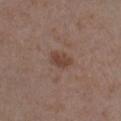- workup: catalogued during a skin exam; not biopsied
- lesion size: ≈2.5 mm
- subject: male, aged around 55
- image: ~15 mm crop, total-body skin-cancer survey
- site: the chest